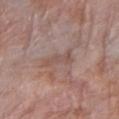biopsy status = no biopsy performed (imaged during a skin exam); subject = female, in their mid- to late 60s; site = the right forearm; image source = total-body-photography crop, ~15 mm field of view.